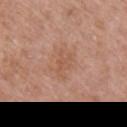biopsy_status: not biopsied; imaged during a skin examination
image:
  source: total-body photography crop
  field_of_view_mm: 15
patient:
  sex: female
  age_approx: 60
site: right upper arm
automated_metrics:
  border_irregularity_0_10: 4.0
  color_variation_0_10: 1.5
  peripheral_color_asymmetry: 0.5
lighting: white-light